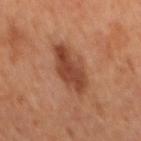Assessment: Captured during whole-body skin photography for melanoma surveillance; the lesion was not biopsied. Clinical summary: A 15 mm close-up tile from a total-body photography series done for melanoma screening. A male patient about 65 years old. On the mid back.